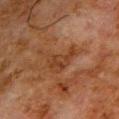Q: Is there a histopathology result?
A: catalogued during a skin exam; not biopsied
Q: How large is the lesion?
A: about 5.5 mm
Q: What are the patient's age and sex?
A: male, aged 78–82
Q: Lesion location?
A: the upper back
Q: What lighting was used for the tile?
A: cross-polarized illumination
Q: Automated lesion metrics?
A: a lesion area of about 11 mm², an eccentricity of roughly 0.7, and two-axis asymmetry of about 0.6; an average lesion color of about L≈31 a*≈18 b*≈28 (CIELAB), about 6 CIELAB-L* units darker than the surrounding skin, and a lesion-to-skin contrast of about 6.5 (normalized; higher = more distinct); a border-irregularity index near 7.5/10 and peripheral color asymmetry of about 1; a classifier nevus-likeness of about 0/100 and lesion-presence confidence of about 100/100
Q: What kind of image is this?
A: ~15 mm tile from a whole-body skin photo